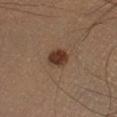Clinical summary: On the right lower leg. Cropped from a whole-body photographic skin survey; the tile spans about 15 mm. An algorithmic analysis of the crop reported border irregularity of about 1.5 on a 0–10 scale and radial color variation of about 1. The analysis additionally found a nevus-likeness score of about 100/100 and lesion-presence confidence of about 100/100. Approximately 3 mm at its widest. The tile uses cross-polarized illumination. A male patient approximately 40 years of age.